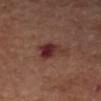biopsy_status: not biopsied; imaged during a skin examination
patient:
  sex: female
  age_approx: 65
lesion_size:
  long_diameter_mm_approx: 3.5
image:
  source: total-body photography crop
  field_of_view_mm: 15
automated_metrics:
  area_mm2_approx: 7.5
  shape_asymmetry: 0.35
  border_irregularity_0_10: 4.0
  color_variation_0_10: 7.0
  peripheral_color_asymmetry: 2.5
site: right forearm